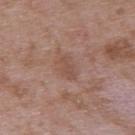* notes · catalogued during a skin exam; not biopsied
* lighting · white-light illumination
* size · about 3 mm
* subject · male, approximately 50 years of age
* body site · the upper back
* acquisition · ~15 mm crop, total-body skin-cancer survey